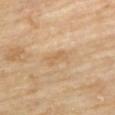| key | value |
|---|---|
| biopsy status | no biopsy performed (imaged during a skin exam) |